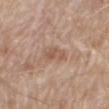workup — imaged on a skin check; not biopsied | automated lesion analysis — a lesion area of about 4.5 mm², a shape eccentricity near 0.85, and a symmetry-axis asymmetry near 0.4; roughly 8 lightness units darker than nearby skin and a lesion-to-skin contrast of about 6 (normalized; higher = more distinct); a within-lesion color-variation index near 3/10 and a peripheral color-asymmetry measure near 1 | tile lighting — white-light illumination | patient — male, aged approximately 80 | image source — total-body-photography crop, ~15 mm field of view | body site — the mid back.